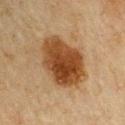Part of a total-body skin-imaging series; this lesion was reviewed on a skin check and was not flagged for biopsy.
The patient is a male aged around 65.
Automated image analysis of the tile measured a mean CIELAB color near L≈35 a*≈18 b*≈31 and about 13 CIELAB-L* units darker than the surrounding skin. And it measured an automated nevus-likeness rating near 100 out of 100 and a lesion-detection confidence of about 100/100.
The lesion is on the right upper arm.
A region of skin cropped from a whole-body photographic capture, roughly 15 mm wide.